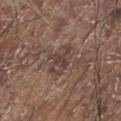Assessment: This lesion was catalogued during total-body skin photography and was not selected for biopsy. Background: A male patient, aged around 80. From the chest. This is a white-light tile. The recorded lesion diameter is about 4.5 mm. An algorithmic analysis of the crop reported a lesion area of about 8.5 mm² and a shape-asymmetry score of about 0.3 (0 = symmetric). Cropped from a whole-body photographic skin survey; the tile spans about 15 mm.